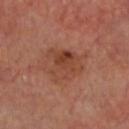  biopsy_status: not biopsied; imaged during a skin examination
  lighting: cross-polarized
  patient:
    sex: male
    age_approx: 65
  lesion_size:
    long_diameter_mm_approx: 4.5
  site: head or neck
  image:
    source: total-body photography crop
    field_of_view_mm: 15